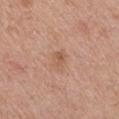Part of a total-body skin-imaging series; this lesion was reviewed on a skin check and was not flagged for biopsy.
The lesion is located on the left upper arm.
The subject is a male roughly 75 years of age.
This is a white-light tile.
Longest diameter approximately 2.5 mm.
Cropped from a total-body skin-imaging series; the visible field is about 15 mm.